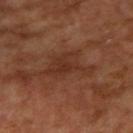  biopsy_status: not biopsied; imaged during a skin examination
  lighting: cross-polarized
  patient:
    sex: male
    age_approx: 55
  site: right upper arm
  lesion_size:
    long_diameter_mm_approx: 7.0
  image:
    source: total-body photography crop
    field_of_view_mm: 15